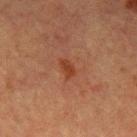biopsy_status: not biopsied; imaged during a skin examination
lighting: cross-polarized
automated_metrics:
  area_mm2_approx: 2.5
  eccentricity: 0.75
  shape_asymmetry: 0.25
  cielab_L: 32
  cielab_a: 22
  cielab_b: 29
  vs_skin_darker_L: 7.0
  vs_skin_contrast_norm: 7.0
lesion_size:
  long_diameter_mm_approx: 2.5
image:
  source: total-body photography crop
  field_of_view_mm: 15
site: left upper arm
patient:
  sex: female
  age_approx: 55